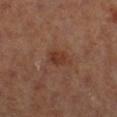biopsy status: imaged on a skin check; not biopsied | site: the right lower leg | illumination: cross-polarized illumination | diameter: about 3.5 mm | subject: female | image source: total-body-photography crop, ~15 mm field of view.